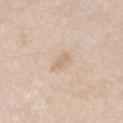Findings:
– notes: imaged on a skin check; not biopsied
– patient: female, in their 60s
– image: 15 mm crop, total-body photography
– site: the right upper arm
– TBP lesion metrics: a lesion area of about 3 mm², an eccentricity of roughly 0.9, and a symmetry-axis asymmetry near 0.3; a border-irregularity index near 3.5/10, a within-lesion color-variation index near 0.5/10, and a peripheral color-asymmetry measure near 0; a detector confidence of about 100 out of 100 that the crop contains a lesion
– lighting: white-light illumination
– lesion diameter: ≈3 mm